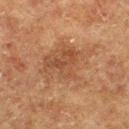Imaged during a routine full-body skin examination; the lesion was not biopsied and no histopathology is available.